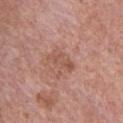follow-up = no biopsy performed (imaged during a skin exam) | TBP lesion metrics = a lesion color around L≈53 a*≈23 b*≈28 in CIELAB and a normalized lesion–skin contrast near 5.5; an automated nevus-likeness rating near 0 out of 100 and a lesion-detection confidence of about 100/100 | image = total-body-photography crop, ~15 mm field of view | patient = male, roughly 70 years of age | site = the chest | lesion diameter = ~3.5 mm (longest diameter).